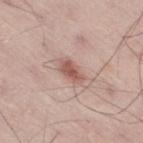Clinical impression:
The lesion was tiled from a total-body skin photograph and was not biopsied.
Acquisition and patient details:
A male subject, in their mid-50s. A region of skin cropped from a whole-body photographic capture, roughly 15 mm wide. Measured at roughly 3.5 mm in maximum diameter. On the right thigh. The tile uses white-light illumination. The lesion-visualizer software estimated a mean CIELAB color near L≈56 a*≈22 b*≈25, roughly 11 lightness units darker than nearby skin, and a normalized border contrast of about 7.5. And it measured a border-irregularity rating of about 2.5/10 and a peripheral color-asymmetry measure near 0.5. It also reported a classifier nevus-likeness of about 70/100 and lesion-presence confidence of about 100/100.